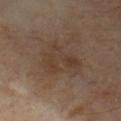Imaged during a routine full-body skin examination; the lesion was not biopsied and no histopathology is available. A male subject, approximately 70 years of age. A lesion tile, about 15 mm wide, cut from a 3D total-body photograph. The tile uses cross-polarized illumination. Longest diameter approximately 5 mm. On the front of the torso.